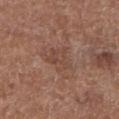follow-up = imaged on a skin check; not biopsied | lighting = white-light | image = ~15 mm crop, total-body skin-cancer survey | image-analysis metrics = a within-lesion color-variation index near 3.5/10 | lesion diameter = ≈3.5 mm | patient = male, in their mid-70s | body site = the left lower leg.